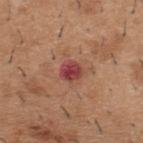Impression: The lesion was photographed on a routine skin check and not biopsied; there is no pathology result. Image and clinical context: A region of skin cropped from a whole-body photographic capture, roughly 15 mm wide. This is a white-light tile. Automated image analysis of the tile measured an outline eccentricity of about 0.45 (0 = round, 1 = elongated) and a shape-asymmetry score of about 0.2 (0 = symmetric). The software also gave an average lesion color of about L≈44 a*≈31 b*≈24 (CIELAB) and a normalized lesion–skin contrast near 10. It also reported a border-irregularity rating of about 1.5/10, internal color variation of about 4 on a 0–10 scale, and radial color variation of about 1.5. A male patient, about 40 years old. From the upper back.